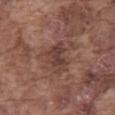Impression:
The lesion was tiled from a total-body skin photograph and was not biopsied.
Image and clinical context:
A male patient aged around 75. Cropped from a whole-body photographic skin survey; the tile spans about 15 mm. From the abdomen. This is a white-light tile. The recorded lesion diameter is about 4 mm.